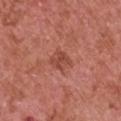Recorded during total-body skin imaging; not selected for excision or biopsy.
This image is a 15 mm lesion crop taken from a total-body photograph.
Automated tile analysis of the lesion measured a footprint of about 4.5 mm² and two-axis asymmetry of about 0.25. The software also gave a mean CIELAB color near L≈47 a*≈28 b*≈29, a lesion–skin lightness drop of about 8, and a lesion-to-skin contrast of about 6 (normalized; higher = more distinct). It also reported a border-irregularity rating of about 2.5/10 and a peripheral color-asymmetry measure near 1. The software also gave lesion-presence confidence of about 100/100.
Imaged with white-light lighting.
Approximately 2.5 mm at its widest.
A male subject aged around 65.
The lesion is on the left upper arm.